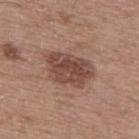– illumination: white-light
– location: the upper back
– subject: male, roughly 60 years of age
– image: ~15 mm tile from a whole-body skin photo